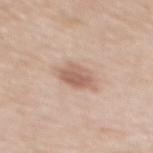No biopsy was performed on this lesion — it was imaged during a full skin examination and was not determined to be concerning.
Cropped from a whole-body photographic skin survey; the tile spans about 15 mm.
Measured at roughly 3.5 mm in maximum diameter.
A male subject aged approximately 70.
Automated image analysis of the tile measured a color-variation rating of about 3/10 and peripheral color asymmetry of about 1.
The lesion is located on the mid back.